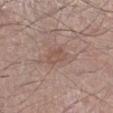Clinical summary: The patient is a male approximately 60 years of age. Cropped from a total-body skin-imaging series; the visible field is about 15 mm. Measured at roughly 3 mm in maximum diameter. The lesion is on the left lower leg.